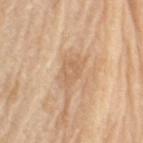Clinical impression: No biopsy was performed on this lesion — it was imaged during a full skin examination and was not determined to be concerning. Acquisition and patient details: The lesion is located on the left upper arm. A 15 mm crop from a total-body photograph taken for skin-cancer surveillance. The patient is a male aged 68–72.